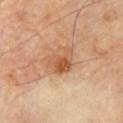| feature | finding |
|---|---|
| workup | total-body-photography surveillance lesion; no biopsy |
| lesion diameter | ≈3.5 mm |
| anatomic site | the chest |
| image-analysis metrics | a lesion color around L≈54 a*≈23 b*≈35 in CIELAB, roughly 10 lightness units darker than nearby skin, and a normalized border contrast of about 7.5; a nevus-likeness score of about 55/100 |
| imaging modality | total-body-photography crop, ~15 mm field of view |
| subject | male, aged around 70 |